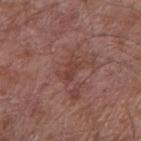Notes:
- notes · total-body-photography surveillance lesion; no biopsy
- lighting · white-light
- acquisition · ~15 mm crop, total-body skin-cancer survey
- lesion diameter · ~2.5 mm (longest diameter)
- body site · the arm
- automated metrics · border irregularity of about 4.5 on a 0–10 scale and a peripheral color-asymmetry measure near 0.5
- patient · male, about 75 years old